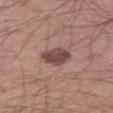Assessment: The lesion was tiled from a total-body skin photograph and was not biopsied. Image and clinical context: The recorded lesion diameter is about 3.5 mm. The tile uses white-light illumination. A male patient about 40 years old. A roughly 15 mm field-of-view crop from a total-body skin photograph. Located on the right thigh.